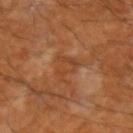<record>
  <biopsy_status>not biopsied; imaged during a skin examination</biopsy_status>
  <automated_metrics>
    <nevus_likeness_0_100>0</nevus_likeness_0_100>
    <lesion_detection_confidence_0_100>95</lesion_detection_confidence_0_100>
  </automated_metrics>
  <lighting>cross-polarized</lighting>
  <image>
    <source>total-body photography crop</source>
    <field_of_view_mm>15</field_of_view_mm>
  </image>
  <site>arm</site>
  <patient>
    <sex>male</sex>
    <age_approx>60</age_approx>
  </patient>
  <lesion_size>
    <long_diameter_mm_approx>3.5</long_diameter_mm_approx>
  </lesion_size>
</record>